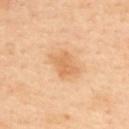<case>
  <biopsy_status>not biopsied; imaged during a skin examination</biopsy_status>
  <patient>
    <sex>female</sex>
    <age_approx>40</age_approx>
  </patient>
  <lighting>cross-polarized</lighting>
  <site>upper back</site>
  <image>
    <source>total-body photography crop</source>
    <field_of_view_mm>15</field_of_view_mm>
  </image>
  <lesion_size>
    <long_diameter_mm_approx>3.5</long_diameter_mm_approx>
  </lesion_size>
  <automated_metrics>
    <area_mm2_approx>7.5</area_mm2_approx>
    <eccentricity>0.65</eccentricity>
    <shape_asymmetry>0.25</shape_asymmetry>
    <border_irregularity_0_10>2.5</border_irregularity_0_10>
    <color_variation_0_10>2.0</color_variation_0_10>
    <peripheral_color_asymmetry>0.5</peripheral_color_asymmetry>
    <nevus_likeness_0_100>20</nevus_likeness_0_100>
    <lesion_detection_confidence_0_100>100</lesion_detection_confidence_0_100>
  </automated_metrics>
</case>